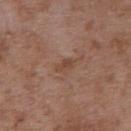biopsy status = catalogued during a skin exam; not biopsied | automated lesion analysis = lesion-presence confidence of about 100/100 | illumination = white-light illumination | diameter = about 2.5 mm | acquisition = total-body-photography crop, ~15 mm field of view | subject = male, approximately 50 years of age | site = the right upper arm.